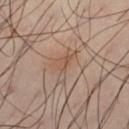The lesion was tiled from a total-body skin photograph and was not biopsied.
From the left thigh.
A region of skin cropped from a whole-body photographic capture, roughly 15 mm wide.
The subject is a male aged 38–42.
Automated tile analysis of the lesion measured a footprint of about 5.5 mm² and a symmetry-axis asymmetry near 0.6. The software also gave an average lesion color of about L≈51 a*≈17 b*≈27 (CIELAB), roughly 6 lightness units darker than nearby skin, and a lesion-to-skin contrast of about 5 (normalized; higher = more distinct). And it measured a border-irregularity index near 7/10, a within-lesion color-variation index near 2/10, and radial color variation of about 1. It also reported a classifier nevus-likeness of about 10/100 and a detector confidence of about 95 out of 100 that the crop contains a lesion.
The recorded lesion diameter is about 4 mm.
This is a cross-polarized tile.